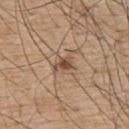Impression: Recorded during total-body skin imaging; not selected for excision or biopsy. Background: A male subject, roughly 55 years of age. The tile uses white-light illumination. The lesion is on the upper back. A 15 mm close-up tile from a total-body photography series done for melanoma screening.